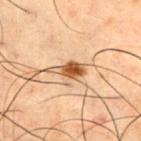Imaged with cross-polarized lighting. A lesion tile, about 15 mm wide, cut from a 3D total-body photograph. A male subject aged around 50. Approximately 3.5 mm at its widest. On the chest.